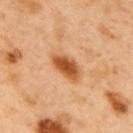The subject is a male aged 48 to 52. Automated tile analysis of the lesion measured a lesion color around L≈55 a*≈26 b*≈42 in CIELAB and roughly 15 lightness units darker than nearby skin. It also reported border irregularity of about 2 on a 0–10 scale and a within-lesion color-variation index near 4/10. The lesion is on the upper back. Captured under cross-polarized illumination. A 15 mm close-up tile from a total-body photography series done for melanoma screening.